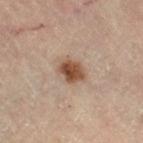Clinical summary:
A lesion tile, about 15 mm wide, cut from a 3D total-body photograph. An algorithmic analysis of the crop reported a lesion area of about 6 mm², an outline eccentricity of about 0.7 (0 = round, 1 = elongated), and a symmetry-axis asymmetry near 0.2. It also reported a lesion color around L≈49 a*≈19 b*≈30 in CIELAB, roughly 14 lightness units darker than nearby skin, and a normalized border contrast of about 10.5. The software also gave a border-irregularity rating of about 2/10, internal color variation of about 5 on a 0–10 scale, and a peripheral color-asymmetry measure near 1.5. It also reported a detector confidence of about 100 out of 100 that the crop contains a lesion. The lesion is on the left thigh. Approximately 3.5 mm at its widest. The patient is a female in their 60s. The tile uses cross-polarized illumination.